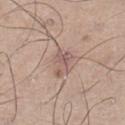  biopsy_status: not biopsied; imaged during a skin examination
  lesion_size:
    long_diameter_mm_approx: 3.5
  patient:
    sex: male
    age_approx: 60
  site: left lower leg
  automated_metrics:
    area_mm2_approx: 5.0
    shape_asymmetry: 0.5
    border_irregularity_0_10: 5.0
    peripheral_color_asymmetry: 1.0
    nevus_likeness_0_100: 0
    lesion_detection_confidence_0_100: 100
  image:
    source: total-body photography crop
    field_of_view_mm: 15